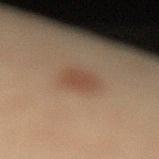Case summary:
• notes · no biopsy performed (imaged during a skin exam)
• patient · female, about 50 years old
• lesion size · about 3 mm
• anatomic site · the left lower leg
• image · total-body-photography crop, ~15 mm field of view